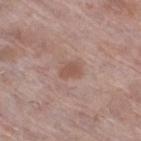- biopsy status: imaged on a skin check; not biopsied
- TBP lesion metrics: an eccentricity of roughly 0.7 and a symmetry-axis asymmetry near 0.2; a mean CIELAB color near L≈53 a*≈19 b*≈26, a lesion–skin lightness drop of about 8, and a normalized border contrast of about 6; border irregularity of about 2 on a 0–10 scale, a color-variation rating of about 1.5/10, and radial color variation of about 0.5; an automated nevus-likeness rating near 15 out of 100
- site: the right thigh
- size: ≈3 mm
- patient: female, roughly 60 years of age
- image source: ~15 mm tile from a whole-body skin photo
- illumination: white-light